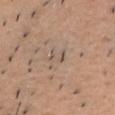Part of a total-body skin-imaging series; this lesion was reviewed on a skin check and was not flagged for biopsy.
The subject is a male about 60 years old.
Cropped from a whole-body photographic skin survey; the tile spans about 15 mm.
On the head or neck.